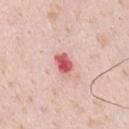The lesion was tiled from a total-body skin photograph and was not biopsied.
Located on the left upper arm.
This is a white-light tile.
A close-up tile cropped from a whole-body skin photograph, about 15 mm across.
The patient is a male approximately 35 years of age.